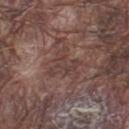<case>
<biopsy_status>not biopsied; imaged during a skin examination</biopsy_status>
<patient>
  <sex>male</sex>
  <age_approx>75</age_approx>
</patient>
<image>
  <source>total-body photography crop</source>
  <field_of_view_mm>15</field_of_view_mm>
</image>
<site>left thigh</site>
</case>